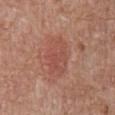No biopsy was performed on this lesion — it was imaged during a full skin examination and was not determined to be concerning. Cropped from a total-body skin-imaging series; the visible field is about 15 mm. Approximately 5 mm at its widest. On the abdomen. The subject is a male aged around 75.